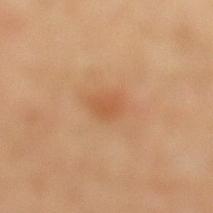Case summary:
- notes · imaged on a skin check; not biopsied
- body site · the left lower leg
- tile lighting · cross-polarized
- image source · ~15 mm crop, total-body skin-cancer survey
- patient · male, aged 63–67
- diameter · ≈2.5 mm